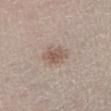biopsy status = total-body-photography surveillance lesion; no biopsy | subject = female, approximately 40 years of age | tile lighting = white-light illumination | location = the right lower leg | imaging modality = total-body-photography crop, ~15 mm field of view | image-analysis metrics = a footprint of about 6 mm² and a symmetry-axis asymmetry near 0.15; an average lesion color of about L≈56 a*≈15 b*≈25 (CIELAB) and a lesion–skin lightness drop of about 9; border irregularity of about 2 on a 0–10 scale, a within-lesion color-variation index near 3/10, and radial color variation of about 1; an automated nevus-likeness rating near 45 out of 100.